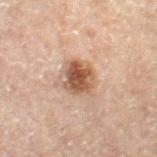Captured during whole-body skin photography for melanoma surveillance; the lesion was not biopsied. A male subject, aged 58 to 62. From the right thigh. A 15 mm crop from a total-body photograph taken for skin-cancer surveillance.